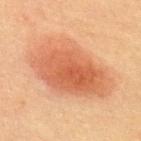The lesion was tiled from a total-body skin photograph and was not biopsied. A lesion tile, about 15 mm wide, cut from a 3D total-body photograph. A male patient, aged 58 to 62. The tile uses cross-polarized illumination. The lesion-visualizer software estimated a footprint of about 43 mm² and a symmetry-axis asymmetry near 0.2. And it measured a lesion color around L≈51 a*≈23 b*≈33 in CIELAB and roughly 10 lightness units darker than nearby skin. And it measured border irregularity of about 2.5 on a 0–10 scale, a color-variation rating of about 5/10, and peripheral color asymmetry of about 1.5. And it measured a nevus-likeness score of about 100/100. Longest diameter approximately 9.5 mm. From the upper back.